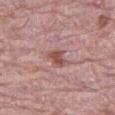biopsy_status: not biopsied; imaged during a skin examination
site: right thigh
lesion_size:
  long_diameter_mm_approx: 2.5
automated_metrics:
  area_mm2_approx: 4.0
  eccentricity: 0.75
  nevus_likeness_0_100: 65
  lesion_detection_confidence_0_100: 100
patient:
  sex: male
  age_approx: 70
image:
  source: total-body photography crop
  field_of_view_mm: 15
lighting: white-light